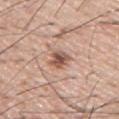| feature | finding |
|---|---|
| follow-up | catalogued during a skin exam; not biopsied |